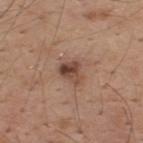Case summary:
* acquisition — ~15 mm crop, total-body skin-cancer survey
* body site — the upper back
* subject — male, aged approximately 40